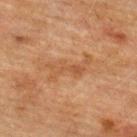Assessment: Imaged during a routine full-body skin examination; the lesion was not biopsied and no histopathology is available. Background: The patient is a male in their mid- to late 70s. Captured under cross-polarized illumination. A 15 mm close-up tile from a total-body photography series done for melanoma screening. The lesion is on the upper back. The lesion-visualizer software estimated a shape eccentricity near 0.9. The analysis additionally found a border-irregularity rating of about 9.5/10, a within-lesion color-variation index near 2.5/10, and peripheral color asymmetry of about 0.5. The recorded lesion diameter is about 5 mm.